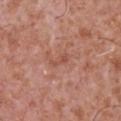This lesion was catalogued during total-body skin photography and was not selected for biopsy.